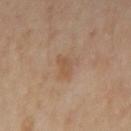| key | value |
|---|---|
| tile lighting | cross-polarized illumination |
| acquisition | total-body-photography crop, ~15 mm field of view |
| patient | female, aged 38–42 |
| automated lesion analysis | an area of roughly 3.5 mm² and an outline eccentricity of about 0.85 (0 = round, 1 = elongated); an average lesion color of about L≈53 a*≈18 b*≈32 (CIELAB), a lesion–skin lightness drop of about 6, and a normalized border contrast of about 5.5; a color-variation rating of about 1.5/10 and peripheral color asymmetry of about 0.5; a classifier nevus-likeness of about 0/100 and a lesion-detection confidence of about 100/100 |
| location | the left forearm |